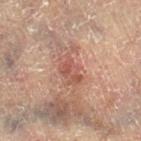Q: Is there a histopathology result?
A: imaged on a skin check; not biopsied
Q: Lesion location?
A: the left leg
Q: What are the patient's age and sex?
A: female, aged approximately 80
Q: What is the lesion's diameter?
A: about 3.5 mm
Q: What is the imaging modality?
A: ~15 mm tile from a whole-body skin photo